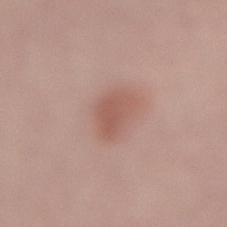{
  "biopsy_status": "not biopsied; imaged during a skin examination",
  "lighting": "white-light",
  "image": {
    "source": "total-body photography crop",
    "field_of_view_mm": 15
  },
  "patient": {
    "sex": "male",
    "age_approx": 70
  },
  "lesion_size": {
    "long_diameter_mm_approx": 3.5
  },
  "site": "back"
}